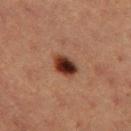Recorded during total-body skin imaging; not selected for excision or biopsy.
A 15 mm close-up tile from a total-body photography series done for melanoma screening.
A female patient approximately 40 years of age.
On the left thigh.